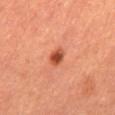automated_metrics:
  border_irregularity_0_10: 2.0
  color_variation_0_10: 3.5
lesion_size:
  long_diameter_mm_approx: 2.5
image:
  source: total-body photography crop
  field_of_view_mm: 15
patient:
  sex: male
  age_approx: 65
site: mid back